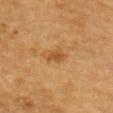biopsy status=total-body-photography surveillance lesion; no biopsy.